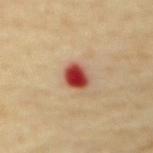follow-up: no biopsy performed (imaged during a skin exam); lesion size: ≈3 mm; subject: male, aged approximately 65; image: 15 mm crop, total-body photography; illumination: cross-polarized; site: the chest.